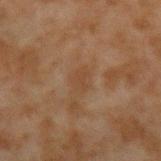  patient:
    sex: male
    age_approx: 45
  image:
    source: total-body photography crop
    field_of_view_mm: 15
  site: arm